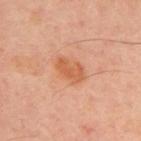site: the upper back | imaging modality: total-body-photography crop, ~15 mm field of view | illumination: cross-polarized | automated lesion analysis: a lesion color around L≈58 a*≈27 b*≈38 in CIELAB and a lesion-to-skin contrast of about 7 (normalized; higher = more distinct); an automated nevus-likeness rating near 40 out of 100 and lesion-presence confidence of about 100/100 | lesion diameter: ≈3.5 mm | patient: male, aged approximately 50.